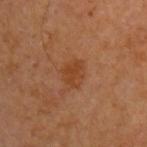Assessment:
Captured during whole-body skin photography for melanoma surveillance; the lesion was not biopsied.
Clinical summary:
From the left upper arm. This image is a 15 mm lesion crop taken from a total-body photograph. A male subject, in their mid- to late 60s.